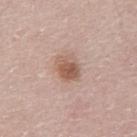| feature | finding |
|---|---|
| notes | no biopsy performed (imaged during a skin exam) |
| patient | male, approximately 65 years of age |
| image source | total-body-photography crop, ~15 mm field of view |
| location | the abdomen |